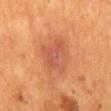Imaged during a routine full-body skin examination; the lesion was not biopsied and no histopathology is available.
A 15 mm close-up tile from a total-body photography series done for melanoma screening.
The tile uses cross-polarized illumination.
Measured at roughly 4 mm in maximum diameter.
The lesion is located on the mid back.
A male patient, roughly 55 years of age.